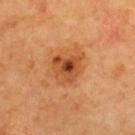Q: Was this lesion biopsied?
A: catalogued during a skin exam; not biopsied
Q: Illumination type?
A: cross-polarized
Q: What is the lesion's diameter?
A: ≈4 mm
Q: Where on the body is the lesion?
A: the upper back
Q: What are the patient's age and sex?
A: approximately 70 years of age
Q: What did automated image analysis measure?
A: a border-irregularity index near 3/10, internal color variation of about 8 on a 0–10 scale, and radial color variation of about 2.5; a nevus-likeness score of about 35/100
Q: How was this image acquired?
A: ~15 mm tile from a whole-body skin photo